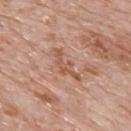This lesion was catalogued during total-body skin photography and was not selected for biopsy. A 15 mm close-up extracted from a 3D total-body photography capture. Captured under white-light illumination. Longest diameter approximately 4.5 mm. A male subject aged around 80. Located on the upper back.